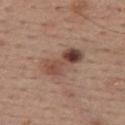The lesion was photographed on a routine skin check and not biopsied; there is no pathology result. A roughly 15 mm field-of-view crop from a total-body skin photograph. A male patient, aged around 55. Imaged with white-light lighting. Located on the back. Measured at roughly 5.5 mm in maximum diameter.